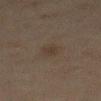body site: the abdomen | acquisition: ~15 mm tile from a whole-body skin photo | lighting: cross-polarized | automated lesion analysis: a lesion area of about 3.5 mm², an outline eccentricity of about 0.85 (0 = round, 1 = elongated), and two-axis asymmetry of about 0.25; a lesion color around L≈28 a*≈9 b*≈19 in CIELAB, about 4 CIELAB-L* units darker than the surrounding skin, and a lesion-to-skin contrast of about 5.5 (normalized; higher = more distinct); a within-lesion color-variation index near 1/10 and peripheral color asymmetry of about 0.5; an automated nevus-likeness rating near 20 out of 100 | patient: male, aged approximately 75 | lesion diameter: ≈3 mm.